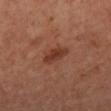<record>
  <biopsy_status>not biopsied; imaged during a skin examination</biopsy_status>
  <lesion_size>
    <long_diameter_mm_approx>3.5</long_diameter_mm_approx>
  </lesion_size>
  <site>leg</site>
  <lighting>cross-polarized</lighting>
  <image>
    <source>total-body photography crop</source>
    <field_of_view_mm>15</field_of_view_mm>
  </image>
  <patient>
    <sex>male</sex>
    <age_approx>65</age_approx>
  </patient>
</record>